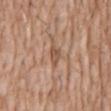  image:
    source: total-body photography crop
    field_of_view_mm: 15
  automated_metrics:
    area_mm2_approx: 3.5
    eccentricity: 0.8
    border_irregularity_0_10: 3.5
    peripheral_color_asymmetry: 1.0
  patient:
    sex: male
    age_approx: 60
  lighting: white-light
  lesion_size:
    long_diameter_mm_approx: 2.5
  site: front of the torso